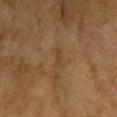Impression: No biopsy was performed on this lesion — it was imaged during a full skin examination and was not determined to be concerning. Image and clinical context: An algorithmic analysis of the crop reported a lesion area of about 2.5 mm², a shape eccentricity near 0.9, and two-axis asymmetry of about 0.45. It also reported about 5 CIELAB-L* units darker than the surrounding skin. And it measured a border-irregularity rating of about 5/10, a color-variation rating of about 0/10, and peripheral color asymmetry of about 0. A 15 mm crop from a total-body photograph taken for skin-cancer surveillance. Measured at roughly 2.5 mm in maximum diameter. Located on the head or neck. Captured under cross-polarized illumination. A female patient aged approximately 60.